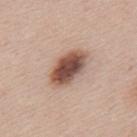No biopsy was performed on this lesion — it was imaged during a full skin examination and was not determined to be concerning. A region of skin cropped from a whole-body photographic capture, roughly 15 mm wide. Located on the back. A male subject, aged 43 to 47.